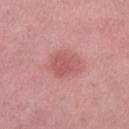biopsy status: catalogued during a skin exam; not biopsied
image: ~15 mm tile from a whole-body skin photo
subject: female, aged 38 to 42
lesion diameter: ≈3.5 mm
anatomic site: the leg
image-analysis metrics: a footprint of about 9 mm², an outline eccentricity of about 0.5 (0 = round, 1 = elongated), and a symmetry-axis asymmetry near 0.25; an average lesion color of about L≈56 a*≈30 b*≈24 (CIELAB) and roughly 8 lightness units darker than nearby skin; a border-irregularity rating of about 2/10, a within-lesion color-variation index near 2/10, and peripheral color asymmetry of about 0.5
lighting: white-light illumination